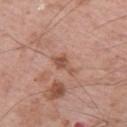biopsy status — imaged on a skin check; not biopsied
patient — male, about 65 years old
acquisition — 15 mm crop, total-body photography
image-analysis metrics — a lesion area of about 4 mm² and two-axis asymmetry of about 0.4; a lesion color around L≈53 a*≈23 b*≈29 in CIELAB, about 10 CIELAB-L* units darker than the surrounding skin, and a normalized lesion–skin contrast near 7
illumination — white-light illumination
body site — the right upper arm